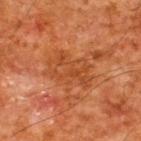– workup — no biopsy performed (imaged during a skin exam)
– site — the back
– image — total-body-photography crop, ~15 mm field of view
– patient — male, aged approximately 60
– TBP lesion metrics — a mean CIELAB color near L≈36 a*≈23 b*≈33, a lesion–skin lightness drop of about 5, and a normalized border contrast of about 5; a border-irregularity rating of about 4.5/10, a within-lesion color-variation index near 3.5/10, and radial color variation of about 1; a nevus-likeness score of about 0/100 and a lesion-detection confidence of about 100/100